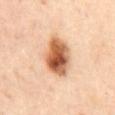This lesion was catalogued during total-body skin photography and was not selected for biopsy.
On the mid back.
A region of skin cropped from a whole-body photographic capture, roughly 15 mm wide.
Imaged with cross-polarized lighting.
The total-body-photography lesion software estimated a lesion area of about 15 mm², an eccentricity of roughly 0.8, and two-axis asymmetry of about 0.2.
Longest diameter approximately 6 mm.
A female subject, roughly 60 years of age.